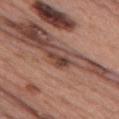workup: no biopsy performed (imaged during a skin exam) | subject: female, aged around 60 | lighting: white-light | anatomic site: the chest | lesion diameter: ~3 mm (longest diameter) | acquisition: total-body-photography crop, ~15 mm field of view | automated metrics: a border-irregularity index near 4.5/10, a color-variation rating of about 2.5/10, and peripheral color asymmetry of about 0.5; an automated nevus-likeness rating near 20 out of 100 and lesion-presence confidence of about 95/100.